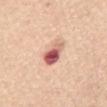Case summary:
• follow-up · total-body-photography surveillance lesion; no biopsy
• patient · female, aged 63 to 67
• site · the abdomen
• image · ~15 mm crop, total-body skin-cancer survey